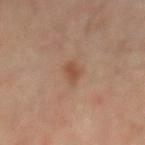follow-up = imaged on a skin check; not biopsied | image source = 15 mm crop, total-body photography | lesion diameter = ~3 mm (longest diameter) | site = the mid back | patient = male, in their mid- to late 60s.